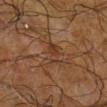Case summary:
– lighting: cross-polarized
– diameter: ~3.5 mm (longest diameter)
– acquisition: total-body-photography crop, ~15 mm field of view
– patient: male, in their mid- to late 60s
– site: the leg